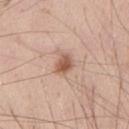Q: Where on the body is the lesion?
A: the front of the torso
Q: What are the patient's age and sex?
A: male, in their mid-40s
Q: How was this image acquired?
A: 15 mm crop, total-body photography
Q: Illumination type?
A: white-light illumination
Q: Lesion size?
A: about 3 mm
Q: What did automated image analysis measure?
A: an area of roughly 4.5 mm², an outline eccentricity of about 0.7 (0 = round, 1 = elongated), and a shape-asymmetry score of about 0.25 (0 = symmetric); a lesion–skin lightness drop of about 12; an automated nevus-likeness rating near 90 out of 100 and a detector confidence of about 100 out of 100 that the crop contains a lesion